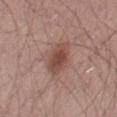Assessment:
Captured during whole-body skin photography for melanoma surveillance; the lesion was not biopsied.
Acquisition and patient details:
The subject is a male in their mid- to late 50s. A roughly 15 mm field-of-view crop from a total-body skin photograph. From the right thigh.